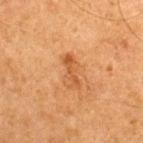Notes:
– workup: no biopsy performed (imaged during a skin exam)
– body site: the upper back
– acquisition: ~15 mm tile from a whole-body skin photo
– lighting: cross-polarized illumination
– automated lesion analysis: an automated nevus-likeness rating near 0 out of 100 and a detector confidence of about 100 out of 100 that the crop contains a lesion
– patient: male, about 65 years old